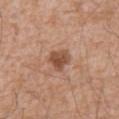Q: What kind of image is this?
A: total-body-photography crop, ~15 mm field of view
Q: What lighting was used for the tile?
A: white-light illumination
Q: Who is the patient?
A: male, aged 58–62
Q: How large is the lesion?
A: ≈3 mm
Q: What did automated image analysis measure?
A: an eccentricity of roughly 0.4 and two-axis asymmetry of about 0.3; a mean CIELAB color near L≈50 a*≈22 b*≈31, about 12 CIELAB-L* units darker than the surrounding skin, and a lesion-to-skin contrast of about 8.5 (normalized; higher = more distinct); a border-irregularity rating of about 2.5/10 and a color-variation rating of about 3.5/10; a nevus-likeness score of about 60/100 and lesion-presence confidence of about 100/100
Q: Where on the body is the lesion?
A: the mid back